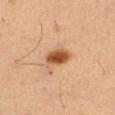– image source · ~15 mm crop, total-body skin-cancer survey
– tile lighting · cross-polarized
– image-analysis metrics · a footprint of about 6.5 mm², an outline eccentricity of about 0.75 (0 = round, 1 = elongated), and a symmetry-axis asymmetry near 0.15; a border-irregularity rating of about 1.5/10, internal color variation of about 4 on a 0–10 scale, and a peripheral color-asymmetry measure near 1
– size · ~3.5 mm (longest diameter)
– anatomic site · the right thigh
– subject · male, aged 48 to 52